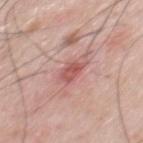No biopsy was performed on this lesion — it was imaged during a full skin examination and was not determined to be concerning.
From the front of the torso.
Automated tile analysis of the lesion measured a border-irregularity rating of about 2/10, a color-variation rating of about 3.5/10, and radial color variation of about 1.5.
A male patient, approximately 60 years of age.
Cropped from a whole-body photographic skin survey; the tile spans about 15 mm.